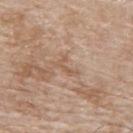Captured during whole-body skin photography for melanoma surveillance; the lesion was not biopsied.
The patient is a male aged 78 to 82.
The tile uses white-light illumination.
A roughly 15 mm field-of-view crop from a total-body skin photograph.
The lesion-visualizer software estimated an automated nevus-likeness rating near 0 out of 100 and lesion-presence confidence of about 90/100.
Measured at roughly 2.5 mm in maximum diameter.
Located on the upper back.